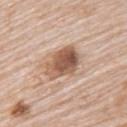biopsy_status: not biopsied; imaged during a skin examination
image:
  source: total-body photography crop
  field_of_view_mm: 15
site: upper back
lesion_size:
  long_diameter_mm_approx: 5.0
patient:
  sex: female
  age_approx: 70
lighting: white-light